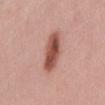Findings:
- follow-up — imaged on a skin check; not biopsied
- automated lesion analysis — a footprint of about 11 mm², a shape eccentricity near 0.9, and a symmetry-axis asymmetry near 0.15; a mean CIELAB color near L≈52 a*≈25 b*≈27, a lesion–skin lightness drop of about 15, and a lesion-to-skin contrast of about 10 (normalized; higher = more distinct); border irregularity of about 2.5 on a 0–10 scale and internal color variation of about 5.5 on a 0–10 scale
- subject — female, approximately 40 years of age
- lesion diameter — ~6 mm (longest diameter)
- anatomic site — the mid back
- imaging modality — ~15 mm tile from a whole-body skin photo